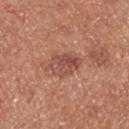workup: imaged on a skin check; not biopsied | acquisition: ~15 mm tile from a whole-body skin photo | lighting: white-light illumination | subject: male, aged around 70 | anatomic site: the right lower leg | size: ≈4.5 mm.